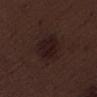biopsy status=imaged on a skin check; not biopsied | image=~15 mm crop, total-body skin-cancer survey | diameter=about 5 mm | anatomic site=the right thigh | illumination=white-light illumination | TBP lesion metrics=a border-irregularity rating of about 2/10, a within-lesion color-variation index near 3.5/10, and a peripheral color-asymmetry measure near 1.5 | patient=male, aged around 70.